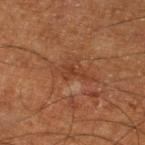No biopsy was performed on this lesion — it was imaged during a full skin examination and was not determined to be concerning. A 15 mm close-up extracted from a 3D total-body photography capture. Located on the left lower leg. Imaged with cross-polarized lighting. The subject is a male in their mid-60s. The recorded lesion diameter is about 3 mm.